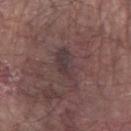Assessment: Imaged during a routine full-body skin examination; the lesion was not biopsied and no histopathology is available. Context: A close-up tile cropped from a whole-body skin photograph, about 15 mm across. The lesion is on the right forearm. A male patient, aged 78 to 82.